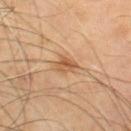Impression: Captured during whole-body skin photography for melanoma surveillance; the lesion was not biopsied. Image and clinical context: Measured at roughly 3 mm in maximum diameter. Automated tile analysis of the lesion measured a border-irregularity index near 3/10, internal color variation of about 4.5 on a 0–10 scale, and radial color variation of about 1.5. The software also gave a lesion-detection confidence of about 100/100. A roughly 15 mm field-of-view crop from a total-body skin photograph. A male subject, aged around 70. The lesion is located on the right thigh. Captured under cross-polarized illumination.